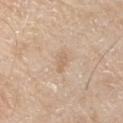Findings:
– workup · catalogued during a skin exam; not biopsied
– body site · the left upper arm
– diameter · ~2.5 mm (longest diameter)
– image source · 15 mm crop, total-body photography
– patient · male, in their mid- to late 60s